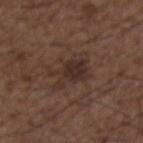follow-up: total-body-photography surveillance lesion; no biopsy | automated metrics: a mean CIELAB color near L≈30 a*≈15 b*≈21, roughly 7 lightness units darker than nearby skin, and a normalized border contrast of about 7.5; border irregularity of about 7 on a 0–10 scale, internal color variation of about 3 on a 0–10 scale, and radial color variation of about 1; a nevus-likeness score of about 15/100 and a detector confidence of about 100 out of 100 that the crop contains a lesion | body site: the chest | patient: male, aged 48 to 52 | image source: ~15 mm tile from a whole-body skin photo | lighting: white-light | diameter: ≈5 mm.